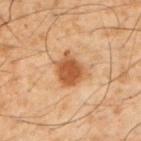Recorded during total-body skin imaging; not selected for excision or biopsy.